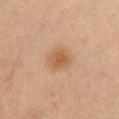<lesion>
  <image>
    <source>total-body photography crop</source>
    <field_of_view_mm>15</field_of_view_mm>
  </image>
  <patient>
    <sex>female</sex>
    <age_approx>40</age_approx>
  </patient>
  <automated_metrics>
    <cielab_L>58</cielab_L>
    <cielab_a>22</cielab_a>
    <cielab_b>37</cielab_b>
    <vs_skin_darker_L>9.0</vs_skin_darker_L>
    <vs_skin_contrast_norm>7.0</vs_skin_contrast_norm>
    <border_irregularity_0_10>2.0</border_irregularity_0_10>
    <color_variation_0_10>3.0</color_variation_0_10>
    <peripheral_color_asymmetry>1.0</peripheral_color_asymmetry>
  </automated_metrics>
  <lighting>cross-polarized</lighting>
  <site>chest</site>
</lesion>